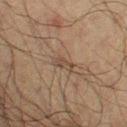Clinical impression:
Recorded during total-body skin imaging; not selected for excision or biopsy.
Image and clinical context:
A 15 mm close-up tile from a total-body photography series done for melanoma screening. On the left upper arm. The tile uses cross-polarized illumination. Approximately 2.5 mm at its widest. A male subject, approximately 70 years of age.